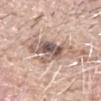biopsy_status: not biopsied; imaged during a skin examination